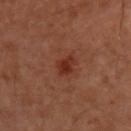Impression:
Part of a total-body skin-imaging series; this lesion was reviewed on a skin check and was not flagged for biopsy.
Context:
Cropped from a whole-body photographic skin survey; the tile spans about 15 mm. Imaged with cross-polarized lighting. A male patient, aged around 50. The lesion is on the upper back. The total-body-photography lesion software estimated an area of roughly 3.5 mm² and a shape eccentricity near 0.75. And it measured a lesion color around L≈31 a*≈27 b*≈30 in CIELAB, about 8 CIELAB-L* units darker than the surrounding skin, and a normalized lesion–skin contrast near 8.5. The software also gave lesion-presence confidence of about 100/100. About 2.5 mm across.